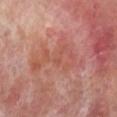The subject is a male in their 70s.
The lesion is on the right lower leg.
A roughly 15 mm field-of-view crop from a total-body skin photograph.
Measured at roughly 7.5 mm in maximum diameter.